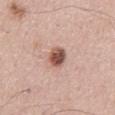No biopsy was performed on this lesion — it was imaged during a full skin examination and was not determined to be concerning. The recorded lesion diameter is about 3 mm. The lesion is on the abdomen. A male patient approximately 55 years of age. The tile uses white-light illumination. A 15 mm close-up tile from a total-body photography series done for melanoma screening.